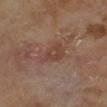Notes:
* notes — total-body-photography surveillance lesion; no biopsy
* patient — male, in their mid-60s
* image — total-body-photography crop, ~15 mm field of view
* site — the right lower leg